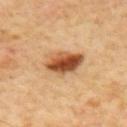The lesion is located on the mid back. Imaged with cross-polarized lighting. The subject is a male aged approximately 55. The recorded lesion diameter is about 4.5 mm. A region of skin cropped from a whole-body photographic capture, roughly 15 mm wide.